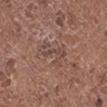<record>
  <site>right lower leg</site>
  <patient>
    <sex>female</sex>
    <age_approx>50</age_approx>
  </patient>
  <image>
    <source>total-body photography crop</source>
    <field_of_view_mm>15</field_of_view_mm>
  </image>
</record>